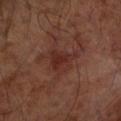Captured during whole-body skin photography for melanoma surveillance; the lesion was not biopsied. The patient is a male in their mid- to late 60s. The lesion is located on the left forearm. Longest diameter approximately 3.5 mm. A lesion tile, about 15 mm wide, cut from a 3D total-body photograph.